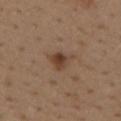Impression: Captured during whole-body skin photography for melanoma surveillance; the lesion was not biopsied. Background: Automated tile analysis of the lesion measured a border-irregularity index near 3.5/10 and radial color variation of about 1.5. And it measured an automated nevus-likeness rating near 90 out of 100 and lesion-presence confidence of about 100/100. The lesion's longest dimension is about 3.5 mm. The lesion is located on the mid back. A close-up tile cropped from a whole-body skin photograph, about 15 mm across. This is a white-light tile. A female patient, in their 40s.